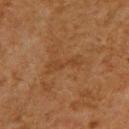Q: Is there a histopathology result?
A: catalogued during a skin exam; not biopsied
Q: Where on the body is the lesion?
A: the upper back
Q: What are the patient's age and sex?
A: male, about 50 years old
Q: What is the imaging modality?
A: total-body-photography crop, ~15 mm field of view